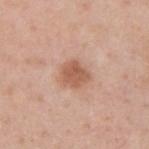{"biopsy_status": "not biopsied; imaged during a skin examination", "image": {"source": "total-body photography crop", "field_of_view_mm": 15}, "patient": {"sex": "male", "age_approx": 40}, "site": "upper back"}